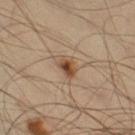The lesion was photographed on a routine skin check and not biopsied; there is no pathology result.
A male subject, aged 33–37.
Approximately 2.5 mm at its widest.
Automated image analysis of the tile measured a footprint of about 3.5 mm², an outline eccentricity of about 0.75 (0 = round, 1 = elongated), and two-axis asymmetry of about 0.25.
Located on the right thigh.
A 15 mm close-up tile from a total-body photography series done for melanoma screening.